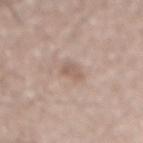Clinical summary:
This is a white-light tile. The patient is a male in their mid-60s. The lesion is located on the lower back. A 15 mm crop from a total-body photograph taken for skin-cancer surveillance. Longest diameter approximately 2.5 mm.